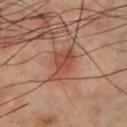Clinical impression:
This lesion was catalogued during total-body skin photography and was not selected for biopsy.